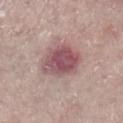Background:
The lesion is on the right lower leg. Automated tile analysis of the lesion measured an area of roughly 15 mm², a shape eccentricity near 0.7, and a symmetry-axis asymmetry near 0.25. The analysis additionally found a lesion color around L≈52 a*≈23 b*≈17 in CIELAB, roughly 13 lightness units darker than nearby skin, and a lesion-to-skin contrast of about 9.5 (normalized; higher = more distinct). A region of skin cropped from a whole-body photographic capture, roughly 15 mm wide. Measured at roughly 5.5 mm in maximum diameter. A female subject approximately 70 years of age.